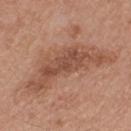- workup — no biopsy performed (imaged during a skin exam)
- automated lesion analysis — a within-lesion color-variation index near 4.5/10
- size — ~11.5 mm (longest diameter)
- imaging modality — ~15 mm crop, total-body skin-cancer survey
- location — the mid back
- patient — male, in their mid- to late 60s